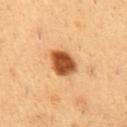The lesion was photographed on a routine skin check and not biopsied; there is no pathology result. An algorithmic analysis of the crop reported an outline eccentricity of about 0.45 (0 = round, 1 = elongated) and a symmetry-axis asymmetry near 0.2. Located on the abdomen. The recorded lesion diameter is about 3.5 mm. A male subject, aged 48 to 52. This is a cross-polarized tile. A region of skin cropped from a whole-body photographic capture, roughly 15 mm wide.